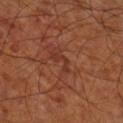Assessment: Imaged during a routine full-body skin examination; the lesion was not biopsied and no histopathology is available. Acquisition and patient details: The lesion is located on the right lower leg. The patient is aged around 65. The tile uses cross-polarized illumination. The lesion-visualizer software estimated an eccentricity of roughly 0.9. The software also gave a normalized border contrast of about 5.5. The software also gave a border-irregularity rating of about 8/10, a within-lesion color-variation index near 0/10, and peripheral color asymmetry of about 0. This image is a 15 mm lesion crop taken from a total-body photograph.